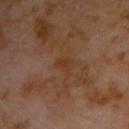follow-up=catalogued during a skin exam; not biopsied | automated metrics=a footprint of about 3 mm², an eccentricity of roughly 0.8, and a symmetry-axis asymmetry near 0.45; a classifier nevus-likeness of about 0/100 and a detector confidence of about 100 out of 100 that the crop contains a lesion | size=~2.5 mm (longest diameter) | subject=male, approximately 60 years of age | location=the right upper arm | image=~15 mm tile from a whole-body skin photo.